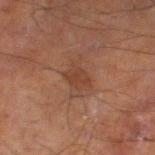Findings:
* notes — total-body-photography surveillance lesion; no biopsy
* automated metrics — a footprint of about 4 mm² and an outline eccentricity of about 0.7 (0 = round, 1 = elongated); roughly 6 lightness units darker than nearby skin and a lesion-to-skin contrast of about 6 (normalized; higher = more distinct); a classifier nevus-likeness of about 0/100 and a detector confidence of about 100 out of 100 that the crop contains a lesion
* lighting — cross-polarized illumination
* subject — male, about 70 years old
* anatomic site — the left lower leg
* acquisition — total-body-photography crop, ~15 mm field of view